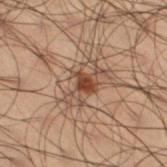workup: no biopsy performed (imaged during a skin exam)
diameter: ~5 mm (longest diameter)
image source: ~15 mm tile from a whole-body skin photo
illumination: cross-polarized
patient: male, about 50 years old
location: the leg